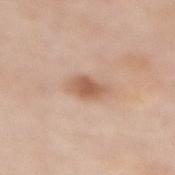  lighting: white-light
  site: back
  automated_metrics:
    area_mm2_approx: 5.5
    eccentricity: 0.8
    shape_asymmetry: 0.15
    cielab_L: 59
    cielab_a: 21
    cielab_b: 31
    vs_skin_darker_L: 12.0
    vs_skin_contrast_norm: 8.0
    border_irregularity_0_10: 2.0
    color_variation_0_10: 2.5
    nevus_likeness_0_100: 75
    lesion_detection_confidence_0_100: 100
  lesion_size:
    long_diameter_mm_approx: 3.5
  patient:
    sex: female
    age_approx: 50
  image:
    source: total-body photography crop
    field_of_view_mm: 15